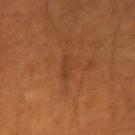Imaged during a routine full-body skin examination; the lesion was not biopsied and no histopathology is available. This is a cross-polarized tile. Cropped from a whole-body photographic skin survey; the tile spans about 15 mm. Measured at roughly 3.5 mm in maximum diameter. Located on the left forearm. The lesion-visualizer software estimated a lesion area of about 3.5 mm², an eccentricity of roughly 0.95, and two-axis asymmetry of about 0.35. It also reported a mean CIELAB color near L≈36 a*≈22 b*≈32, a lesion–skin lightness drop of about 5, and a lesion-to-skin contrast of about 5 (normalized; higher = more distinct). The analysis additionally found an automated nevus-likeness rating near 0 out of 100. A male patient, in their 60s.